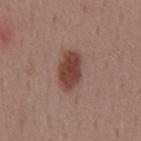Impression: This lesion was catalogued during total-body skin photography and was not selected for biopsy. Image and clinical context: Cropped from a whole-body photographic skin survey; the tile spans about 15 mm. A male patient, in their mid- to late 50s. The lesion is located on the mid back.